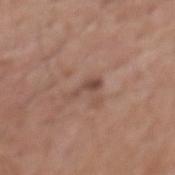Assessment: The lesion was photographed on a routine skin check and not biopsied; there is no pathology result. Context: Located on the back. The subject is a male about 75 years old. Measured at roughly 3 mm in maximum diameter. Cropped from a whole-body photographic skin survey; the tile spans about 15 mm.